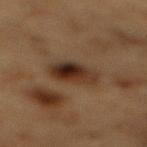Clinical impression: The lesion was photographed on a routine skin check and not biopsied; there is no pathology result. Image and clinical context: A roughly 15 mm field-of-view crop from a total-body skin photograph. Located on the mid back. Imaged with cross-polarized lighting. The lesion's longest dimension is about 4.5 mm. The patient is a male aged 83 or older.